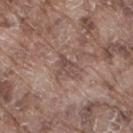This lesion was catalogued during total-body skin photography and was not selected for biopsy.
Approximately 2.5 mm at its widest.
A male subject aged 73 to 77.
The lesion is on the right thigh.
Cropped from a whole-body photographic skin survey; the tile spans about 15 mm.